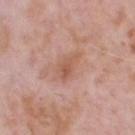Impression: Recorded during total-body skin imaging; not selected for excision or biopsy. Context: Captured under white-light illumination. The lesion is located on the head or neck. A roughly 15 mm field-of-view crop from a total-body skin photograph. A male patient in their 70s.